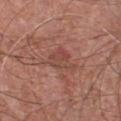The tile uses white-light illumination. A male patient, aged 63 to 67. This image is a 15 mm lesion crop taken from a total-body photograph. The lesion is on the chest. Measured at roughly 4.5 mm in maximum diameter.